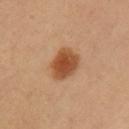The lesion was tiled from a total-body skin photograph and was not biopsied. Imaged with cross-polarized lighting. An algorithmic analysis of the crop reported a border-irregularity index near 1.5/10 and a peripheral color-asymmetry measure near 1. The lesion is located on the left upper arm. Cropped from a whole-body photographic skin survey; the tile spans about 15 mm. A female subject, in their 40s. Approximately 4 mm at its widest.